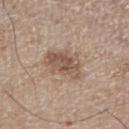biopsy status: catalogued during a skin exam; not biopsied
size: about 5 mm
body site: the right lower leg
image source: total-body-photography crop, ~15 mm field of view
subject: male, about 65 years old
TBP lesion metrics: a mean CIELAB color near L≈54 a*≈16 b*≈26, roughly 10 lightness units darker than nearby skin, and a normalized lesion–skin contrast near 7; border irregularity of about 3.5 on a 0–10 scale, a color-variation rating of about 4.5/10, and a peripheral color-asymmetry measure near 2
tile lighting: white-light